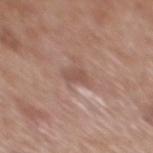Clinical summary:
Imaged with white-light lighting. A 15 mm close-up extracted from a 3D total-body photography capture. The patient is a male roughly 70 years of age. The lesion is on the mid back.